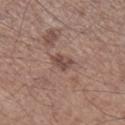Q: What is the lesion's diameter?
A: ~3 mm (longest diameter)
Q: What is the anatomic site?
A: the right lower leg
Q: How was this image acquired?
A: ~15 mm crop, total-body skin-cancer survey
Q: Who is the patient?
A: male, aged approximately 65
Q: What did automated image analysis measure?
A: an area of roughly 4 mm² and an eccentricity of roughly 0.75; a lesion color around L≈46 a*≈18 b*≈23 in CIELAB, roughly 10 lightness units darker than nearby skin, and a normalized border contrast of about 7.5; internal color variation of about 3 on a 0–10 scale and peripheral color asymmetry of about 1; an automated nevus-likeness rating near 0 out of 100 and lesion-presence confidence of about 100/100
Q: Illumination type?
A: white-light illumination